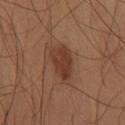No biopsy was performed on this lesion — it was imaged during a full skin examination and was not determined to be concerning. From the right thigh. A male patient, aged 58 to 62. A 15 mm crop from a total-body photograph taken for skin-cancer surveillance.